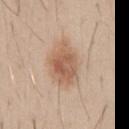{"biopsy_status": "not biopsied; imaged during a skin examination", "lighting": "white-light", "automated_metrics": {"area_mm2_approx": 14.0, "eccentricity": 0.75, "shape_asymmetry": 0.2}, "site": "abdomen", "image": {"source": "total-body photography crop", "field_of_view_mm": 15}, "lesion_size": {"long_diameter_mm_approx": 5.5}, "patient": {"sex": "male", "age_approx": 30}}